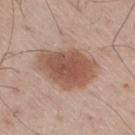Q: Was this lesion biopsied?
A: imaged on a skin check; not biopsied
Q: Illumination type?
A: white-light illumination
Q: What kind of image is this?
A: total-body-photography crop, ~15 mm field of view
Q: What are the patient's age and sex?
A: male, approximately 60 years of age
Q: Where on the body is the lesion?
A: the right thigh